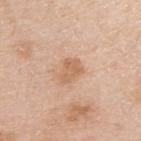workup: no biopsy performed (imaged during a skin exam)
acquisition: total-body-photography crop, ~15 mm field of view
location: the right upper arm
tile lighting: white-light
patient: female, in their mid- to late 40s